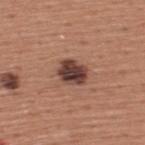This lesion was catalogued during total-body skin photography and was not selected for biopsy. Approximately 3.5 mm at its widest. On the upper back. Cropped from a total-body skin-imaging series; the visible field is about 15 mm. A male patient in their mid- to late 40s. The tile uses white-light illumination.